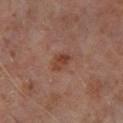Notes:
• biopsy status · no biopsy performed (imaged during a skin exam)
• site · the leg
• imaging modality · ~15 mm tile from a whole-body skin photo
• patient · male, approximately 60 years of age
• lesion size · ~2.5 mm (longest diameter)